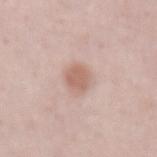{"biopsy_status": "not biopsied; imaged during a skin examination", "lesion_size": {"long_diameter_mm_approx": 3.5}, "image": {"source": "total-body photography crop", "field_of_view_mm": 15}, "automated_metrics": {"nevus_likeness_0_100": 90, "lesion_detection_confidence_0_100": 100}, "site": "abdomen", "lighting": "white-light", "patient": {"sex": "male", "age_approx": 60}}